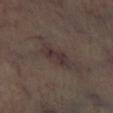Clinical impression: This lesion was catalogued during total-body skin photography and was not selected for biopsy. Acquisition and patient details: Located on the left lower leg. The tile uses cross-polarized illumination. Approximately 4 mm at its widest. A close-up tile cropped from a whole-body skin photograph, about 15 mm across.